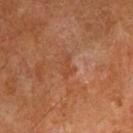Case summary:
* biopsy status · imaged on a skin check; not biopsied
* patient · male, aged around 60
* site · the right upper arm
* imaging modality · ~15 mm crop, total-body skin-cancer survey